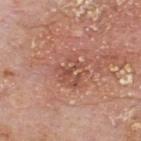Recorded during total-body skin imaging; not selected for excision or biopsy. A 15 mm close-up extracted from a 3D total-body photography capture. The recorded lesion diameter is about 2.5 mm. Automated image analysis of the tile measured an area of roughly 2 mm² and a shape eccentricity near 0.9. The patient is a male in their mid- to late 70s. The lesion is on the back.